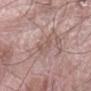No biopsy was performed on this lesion — it was imaged during a full skin examination and was not determined to be concerning.
On the left forearm.
A close-up tile cropped from a whole-body skin photograph, about 15 mm across.
This is a white-light tile.
The subject is a male aged 58–62.
Approximately 2.5 mm at its widest.